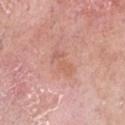{
  "biopsy_status": "not biopsied; imaged during a skin examination",
  "automated_metrics": {
    "area_mm2_approx": 5.0,
    "eccentricity": 0.9,
    "shape_asymmetry": 0.3
  },
  "lesion_size": {
    "long_diameter_mm_approx": 3.5
  },
  "site": "chest",
  "patient": {
    "sex": "female",
    "age_approx": 70
  },
  "image": {
    "source": "total-body photography crop",
    "field_of_view_mm": 15
  },
  "lighting": "white-light"
}